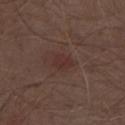location: the chest; image source: 15 mm crop, total-body photography; subject: male, about 70 years old; lighting: white-light; lesion size: about 2.5 mm.